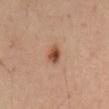Clinical impression:
Recorded during total-body skin imaging; not selected for excision or biopsy.
Clinical summary:
A male patient in their mid- to late 50s. This is a cross-polarized tile. This image is a 15 mm lesion crop taken from a total-body photograph. Measured at roughly 2.5 mm in maximum diameter. From the back.